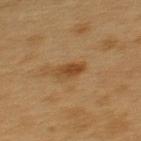Assessment:
Part of a total-body skin-imaging series; this lesion was reviewed on a skin check and was not flagged for biopsy.
Acquisition and patient details:
The tile uses cross-polarized illumination. A lesion tile, about 15 mm wide, cut from a 3D total-body photograph. The lesion is located on the upper back. A male patient approximately 55 years of age. The recorded lesion diameter is about 3 mm. Automated image analysis of the tile measured an average lesion color of about L≈40 a*≈17 b*≈34 (CIELAB), a lesion–skin lightness drop of about 9, and a lesion-to-skin contrast of about 7.5 (normalized; higher = more distinct). It also reported a border-irregularity index near 2/10, internal color variation of about 3 on a 0–10 scale, and radial color variation of about 1.